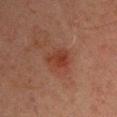No biopsy was performed on this lesion — it was imaged during a full skin examination and was not determined to be concerning. The recorded lesion diameter is about 3.5 mm. A male patient in their mid-40s. The lesion-visualizer software estimated a lesion area of about 7.5 mm², an eccentricity of roughly 0.65, and two-axis asymmetry of about 0.2. It also reported an average lesion color of about L≈32 a*≈22 b*≈25 (CIELAB), a lesion–skin lightness drop of about 7, and a lesion-to-skin contrast of about 7 (normalized; higher = more distinct). And it measured a border-irregularity index near 2/10, internal color variation of about 4 on a 0–10 scale, and a peripheral color-asymmetry measure near 1.5. A region of skin cropped from a whole-body photographic capture, roughly 15 mm wide. This is a cross-polarized tile. The lesion is located on the arm.